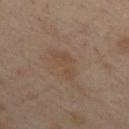Q: Was a biopsy performed?
A: catalogued during a skin exam; not biopsied
Q: Illumination type?
A: cross-polarized
Q: What did automated image analysis measure?
A: an area of roughly 4.5 mm² and a shape eccentricity near 0.9; roughly 4 lightness units darker than nearby skin and a normalized border contrast of about 4.5; a nevus-likeness score of about 0/100
Q: Where on the body is the lesion?
A: the chest
Q: Who is the patient?
A: female, roughly 55 years of age
Q: Lesion size?
A: ≈3.5 mm
Q: How was this image acquired?
A: 15 mm crop, total-body photography